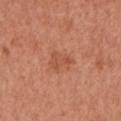Notes:
- notes — total-body-photography surveillance lesion; no biopsy
- image source — total-body-photography crop, ~15 mm field of view
- location — the left upper arm
- diameter — ~3 mm (longest diameter)
- patient — male, aged 63 to 67